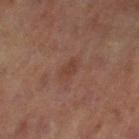Q: Was a biopsy performed?
A: total-body-photography surveillance lesion; no biopsy
Q: Lesion location?
A: the left thigh
Q: What kind of image is this?
A: total-body-photography crop, ~15 mm field of view
Q: Automated lesion metrics?
A: an average lesion color of about L≈35 a*≈18 b*≈23 (CIELAB), a lesion–skin lightness drop of about 5, and a lesion-to-skin contrast of about 5 (normalized; higher = more distinct); a lesion-detection confidence of about 100/100
Q: Illumination type?
A: cross-polarized illumination
Q: Who is the patient?
A: female, aged around 60